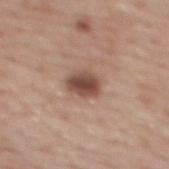Assessment:
The lesion was tiled from a total-body skin photograph and was not biopsied.
Acquisition and patient details:
Located on the upper back. An algorithmic analysis of the crop reported a lesion area of about 6 mm², an eccentricity of roughly 0.65, and two-axis asymmetry of about 0.2. The software also gave a mean CIELAB color near L≈47 a*≈20 b*≈25 and a lesion–skin lightness drop of about 14. The software also gave a border-irregularity rating of about 1.5/10 and a peripheral color-asymmetry measure near 1. The software also gave a nevus-likeness score of about 90/100 and lesion-presence confidence of about 100/100. Imaged with white-light lighting. The lesion's longest dimension is about 3 mm. A close-up tile cropped from a whole-body skin photograph, about 15 mm across. A female patient, aged approximately 60.